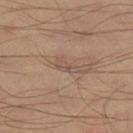Background: This is a cross-polarized tile. A male patient aged 33–37. Cropped from a total-body skin-imaging series; the visible field is about 15 mm. The lesion is located on the leg.